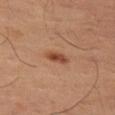Recorded during total-body skin imaging; not selected for excision or biopsy.
Cropped from a whole-body photographic skin survey; the tile spans about 15 mm.
Longest diameter approximately 3.5 mm.
A male subject roughly 50 years of age.
On the left thigh.
Imaged with cross-polarized lighting.